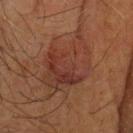follow-up = catalogued during a skin exam; not biopsied | acquisition = total-body-photography crop, ~15 mm field of view | site = the head or neck | lesion size = about 9 mm | patient = male, aged approximately 65.